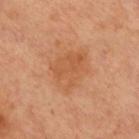{"patient": {"sex": "female", "age_approx": 55}, "image": {"source": "total-body photography crop", "field_of_view_mm": 15}, "site": "front of the torso"}